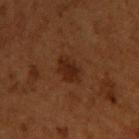No biopsy was performed on this lesion — it was imaged during a full skin examination and was not determined to be concerning.
The lesion is on the chest.
The patient is a male aged approximately 50.
Captured under cross-polarized illumination.
A close-up tile cropped from a whole-body skin photograph, about 15 mm across.
Approximately 4 mm at its widest.
The lesion-visualizer software estimated a lesion area of about 6.5 mm², an outline eccentricity of about 0.85 (0 = round, 1 = elongated), and a shape-asymmetry score of about 0.15 (0 = symmetric). And it measured a lesion color around L≈19 a*≈17 b*≈23 in CIELAB, roughly 6 lightness units darker than nearby skin, and a lesion-to-skin contrast of about 7.5 (normalized; higher = more distinct). It also reported a within-lesion color-variation index near 2/10 and peripheral color asymmetry of about 0.5. The analysis additionally found a nevus-likeness score of about 60/100 and a lesion-detection confidence of about 100/100.